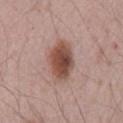{
  "biopsy_status": "not biopsied; imaged during a skin examination",
  "lesion_size": {
    "long_diameter_mm_approx": 5.0
  },
  "lighting": "white-light",
  "image": {
    "source": "total-body photography crop",
    "field_of_view_mm": 15
  },
  "automated_metrics": {
    "area_mm2_approx": 14.0,
    "cielab_L": 49,
    "cielab_a": 21,
    "cielab_b": 25,
    "vs_skin_darker_L": 14.0,
    "lesion_detection_confidence_0_100": 100
  },
  "patient": {
    "sex": "male",
    "age_approx": 65
  },
  "site": "mid back"
}